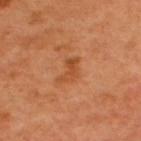The lesion was tiled from a total-body skin photograph and was not biopsied. The lesion is located on the upper back. A male subject aged around 50. The total-body-photography lesion software estimated a color-variation rating of about 1.5/10 and radial color variation of about 0. The software also gave an automated nevus-likeness rating near 5 out of 100 and a lesion-detection confidence of about 100/100. Longest diameter approximately 3.5 mm. A 15 mm close-up extracted from a 3D total-body photography capture.